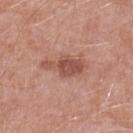Q: Was this lesion biopsied?
A: total-body-photography surveillance lesion; no biopsy
Q: What kind of image is this?
A: ~15 mm crop, total-body skin-cancer survey
Q: Who is the patient?
A: male, approximately 50 years of age
Q: Lesion size?
A: ~5 mm (longest diameter)
Q: What is the anatomic site?
A: the left lower leg